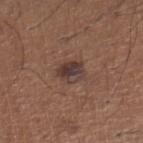notes: catalogued during a skin exam; not biopsied
illumination: white-light
imaging modality: total-body-photography crop, ~15 mm field of view
subject: male, aged approximately 65
lesion size: about 3 mm
site: the right lower leg
TBP lesion metrics: a lesion area of about 6.5 mm², an outline eccentricity of about 0.55 (0 = round, 1 = elongated), and a symmetry-axis asymmetry near 0.2; a border-irregularity index near 2/10 and a within-lesion color-variation index near 5/10; a detector confidence of about 100 out of 100 that the crop contains a lesion